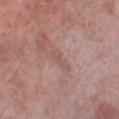Automated image analysis of the tile measured a lesion area of about 3 mm², an eccentricity of roughly 0.95, and a shape-asymmetry score of about 0.35 (0 = symmetric). The software also gave an average lesion color of about L≈54 a*≈20 b*≈24 (CIELAB) and a normalized border contrast of about 5. A male patient roughly 55 years of age. Cropped from a whole-body photographic skin survey; the tile spans about 15 mm. About 3 mm across. From the left lower leg.